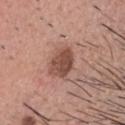| field | value |
|---|---|
| follow-up | catalogued during a skin exam; not biopsied |
| body site | the head or neck |
| lesion size | ~3.5 mm (longest diameter) |
| patient | male, roughly 35 years of age |
| TBP lesion metrics | a lesion area of about 8.5 mm², a shape eccentricity near 0.5, and two-axis asymmetry of about 0.15; a border-irregularity rating of about 1.5/10, a color-variation rating of about 3.5/10, and radial color variation of about 1 |
| imaging modality | total-body-photography crop, ~15 mm field of view |